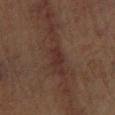Q: Was a biopsy performed?
A: no biopsy performed (imaged during a skin exam)
Q: Where on the body is the lesion?
A: the leg
Q: How was the tile lit?
A: cross-polarized
Q: How was this image acquired?
A: 15 mm crop, total-body photography
Q: Patient demographics?
A: female, aged around 65